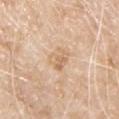Assessment:
Captured during whole-body skin photography for melanoma surveillance; the lesion was not biopsied.
Clinical summary:
The recorded lesion diameter is about 3 mm. A male subject aged approximately 80. Automated image analysis of the tile measured a mean CIELAB color near L≈67 a*≈17 b*≈35, roughly 8 lightness units darker than nearby skin, and a lesion-to-skin contrast of about 5.5 (normalized; higher = more distinct). The software also gave a within-lesion color-variation index near 4/10 and peripheral color asymmetry of about 1.5. A close-up tile cropped from a whole-body skin photograph, about 15 mm across. The lesion is located on the front of the torso. The tile uses white-light illumination.